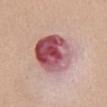  biopsy_status: not biopsied; imaged during a skin examination
  patient:
    sex: male
    age_approx: 35
  image:
    source: total-body photography crop
    field_of_view_mm: 15
  lesion_size:
    long_diameter_mm_approx: 7.0
  site: chest
  lighting: white-light